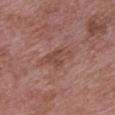notes = imaged on a skin check; not biopsied.